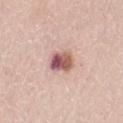Recorded during total-body skin imaging; not selected for excision or biopsy.
The lesion is on the left thigh.
The lesion-visualizer software estimated an average lesion color of about L≈57 a*≈25 b*≈24 (CIELAB), roughly 17 lightness units darker than nearby skin, and a normalized lesion–skin contrast near 10.5. And it measured internal color variation of about 10 on a 0–10 scale and a peripheral color-asymmetry measure near 5. The analysis additionally found lesion-presence confidence of about 100/100.
Captured under white-light illumination.
A 15 mm close-up tile from a total-body photography series done for melanoma screening.
A female patient, aged around 65.